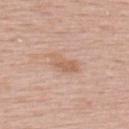Findings:
- lighting: white-light
- location: the upper back
- image source: total-body-photography crop, ~15 mm field of view
- patient: male, in their mid-50s
- automated metrics: a lesion area of about 6 mm², an outline eccentricity of about 0.85 (0 = round, 1 = elongated), and a symmetry-axis asymmetry near 0.35; a classifier nevus-likeness of about 0/100 and a detector confidence of about 100 out of 100 that the crop contains a lesion